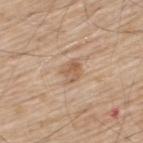follow-up: imaged on a skin check; not biopsied | image-analysis metrics: a mean CIELAB color near L≈58 a*≈18 b*≈32 and roughly 9 lightness units darker than nearby skin; a border-irregularity index near 2/10 and internal color variation of about 4 on a 0–10 scale | lesion size: ≈2.5 mm | site: the upper back | acquisition: ~15 mm crop, total-body skin-cancer survey | patient: male, approximately 70 years of age.